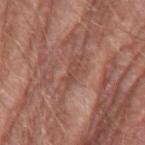<record>
  <biopsy_status>not biopsied; imaged during a skin examination</biopsy_status>
  <lighting>white-light</lighting>
  <automated_metrics>
    <cielab_L>47</cielab_L>
    <cielab_a>23</cielab_a>
    <cielab_b>25</cielab_b>
    <vs_skin_darker_L>7.0</vs_skin_darker_L>
    <vs_skin_contrast_norm>5.5</vs_skin_contrast_norm>
    <lesion_detection_confidence_0_100>55</lesion_detection_confidence_0_100>
  </automated_metrics>
  <site>left upper arm</site>
  <image>
    <source>total-body photography crop</source>
    <field_of_view_mm>15</field_of_view_mm>
  </image>
  <lesion_size>
    <long_diameter_mm_approx>2.5</long_diameter_mm_approx>
  </lesion_size>
  <patient>
    <sex>male</sex>
    <age_approx>80</age_approx>
  </patient>
</record>